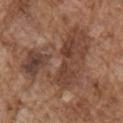Findings:
• image-analysis metrics: a border-irregularity rating of about 10/10, internal color variation of about 6.5 on a 0–10 scale, and a peripheral color-asymmetry measure near 2.5; a classifier nevus-likeness of about 0/100 and a lesion-detection confidence of about 90/100
• anatomic site: the right upper arm
• diameter: ≈9.5 mm
• subject: male, about 75 years old
• acquisition: total-body-photography crop, ~15 mm field of view